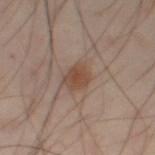The lesion was photographed on a routine skin check and not biopsied; there is no pathology result. This is a cross-polarized tile. A male patient in their mid- to late 50s. About 3 mm across. A roughly 15 mm field-of-view crop from a total-body skin photograph. The lesion is located on the leg.